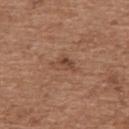biopsy status: total-body-photography surveillance lesion; no biopsy